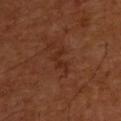Notes:
• biopsy status: no biopsy performed (imaged during a skin exam)
• automated lesion analysis: a classifier nevus-likeness of about 0/100
• subject: male, about 55 years old
• size: ~3 mm (longest diameter)
• acquisition: ~15 mm tile from a whole-body skin photo
• anatomic site: the upper back
• lighting: cross-polarized